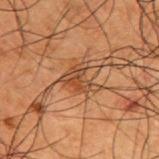Q: Is there a histopathology result?
A: total-body-photography surveillance lesion; no biopsy
Q: What is the imaging modality?
A: ~15 mm tile from a whole-body skin photo
Q: What is the anatomic site?
A: the upper back
Q: Illumination type?
A: cross-polarized illumination
Q: Patient demographics?
A: male, about 50 years old
Q: What is the lesion's diameter?
A: ~4 mm (longest diameter)